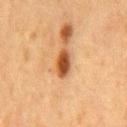site: the mid back | lesion diameter: ≈3.5 mm | illumination: cross-polarized | subject: male, aged around 75 | acquisition: ~15 mm crop, total-body skin-cancer survey | image-analysis metrics: an average lesion color of about L≈42 a*≈23 b*≈34 (CIELAB) and about 14 CIELAB-L* units darker than the surrounding skin; a border-irregularity index near 1.5/10, a color-variation rating of about 4.5/10, and peripheral color asymmetry of about 1.5.